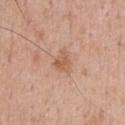Captured during whole-body skin photography for melanoma surveillance; the lesion was not biopsied. Imaged with white-light lighting. An algorithmic analysis of the crop reported an average lesion color of about L≈58 a*≈21 b*≈32 (CIELAB), about 8 CIELAB-L* units darker than the surrounding skin, and a lesion-to-skin contrast of about 6 (normalized; higher = more distinct). The software also gave a color-variation rating of about 2.5/10 and peripheral color asymmetry of about 1. The software also gave an automated nevus-likeness rating near 0 out of 100. About 2.5 mm across. A male patient, aged approximately 55. The lesion is located on the chest. A 15 mm close-up extracted from a 3D total-body photography capture.